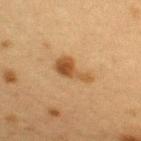notes: total-body-photography surveillance lesion; no biopsy
location: the right upper arm
patient: female, approximately 40 years of age
illumination: cross-polarized illumination
image: total-body-photography crop, ~15 mm field of view
TBP lesion metrics: a lesion area of about 7.5 mm² and a shape eccentricity near 0.9